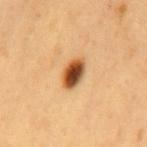– biopsy status · no biopsy performed (imaged during a skin exam)
– site · the mid back
– image source · 15 mm crop, total-body photography
– subject · male, roughly 60 years of age
– image-analysis metrics · an area of roughly 6.5 mm², a shape eccentricity near 0.8, and a symmetry-axis asymmetry near 0.25; a border-irregularity rating of about 2/10, a color-variation rating of about 8/10, and radial color variation of about 2; an automated nevus-likeness rating near 100 out of 100 and a detector confidence of about 100 out of 100 that the crop contains a lesion
– lesion size · ~3.5 mm (longest diameter)